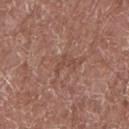Captured under white-light illumination. Automated image analysis of the tile measured a lesion area of about 2 mm². The analysis additionally found a normalized border contrast of about 5. It also reported a detector confidence of about 70 out of 100 that the crop contains a lesion. Located on the arm. Longest diameter approximately 3 mm. Cropped from a total-body skin-imaging series; the visible field is about 15 mm. A male patient aged 68 to 72.